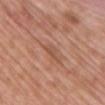subject — female, aged approximately 65; anatomic site — the chest; illumination — white-light illumination; image source — 15 mm crop, total-body photography; automated lesion analysis — a border-irregularity index near 4.5/10, a color-variation rating of about 2/10, and peripheral color asymmetry of about 0.5; lesion diameter — ≈3 mm.